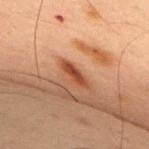The recorded lesion diameter is about 3.5 mm.
Automated image analysis of the tile measured an eccentricity of roughly 0.95.
Imaged with cross-polarized lighting.
The lesion is on the upper back.
A male patient, in their 60s.
Cropped from a total-body skin-imaging series; the visible field is about 15 mm.